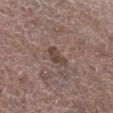{
  "biopsy_status": "not biopsied; imaged during a skin examination",
  "patient": {
    "sex": "male",
    "age_approx": 70
  },
  "site": "right lower leg",
  "image": {
    "source": "total-body photography crop",
    "field_of_view_mm": 15
  }
}